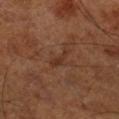The lesion was photographed on a routine skin check and not biopsied; there is no pathology result.
Measured at roughly 3 mm in maximum diameter.
The patient is in their mid- to late 60s.
Imaged with cross-polarized lighting.
A 15 mm crop from a total-body photograph taken for skin-cancer surveillance.
Automated tile analysis of the lesion measured an eccentricity of roughly 0.9 and a symmetry-axis asymmetry near 0.5. The software also gave an average lesion color of about L≈33 a*≈20 b*≈27 (CIELAB), a lesion–skin lightness drop of about 6, and a normalized border contrast of about 5.5. It also reported a nevus-likeness score of about 0/100 and a lesion-detection confidence of about 100/100.
From the right lower leg.